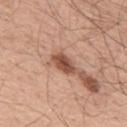{
  "biopsy_status": "not biopsied; imaged during a skin examination",
  "automated_metrics": {
    "area_mm2_approx": 4.5,
    "eccentricity": 0.8,
    "shape_asymmetry": 0.2,
    "vs_skin_darker_L": 15.0,
    "border_irregularity_0_10": 2.0,
    "color_variation_0_10": 3.0,
    "peripheral_color_asymmetry": 1.0,
    "lesion_detection_confidence_0_100": 100
  },
  "site": "upper back",
  "patient": {
    "sex": "male",
    "age_approx": 55
  },
  "lesion_size": {
    "long_diameter_mm_approx": 3.0
  },
  "lighting": "white-light",
  "image": {
    "source": "total-body photography crop",
    "field_of_view_mm": 15
  }
}